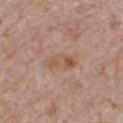{"biopsy_status": "not biopsied; imaged during a skin examination", "automated_metrics": {"color_variation_0_10": 4.5, "peripheral_color_asymmetry": 1.5}, "lesion_size": {"long_diameter_mm_approx": 5.0}, "site": "chest", "patient": {"sex": "male", "age_approx": 70}, "lighting": "white-light", "image": {"source": "total-body photography crop", "field_of_view_mm": 15}}